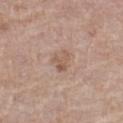Assessment:
Imaged during a routine full-body skin examination; the lesion was not biopsied and no histopathology is available.
Clinical summary:
Longest diameter approximately 3 mm. Captured under white-light illumination. A 15 mm crop from a total-body photograph taken for skin-cancer surveillance. A male patient, aged 78 to 82. Located on the left thigh.